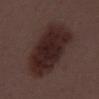Recorded during total-body skin imaging; not selected for excision or biopsy. A male subject, aged around 55. The recorded lesion diameter is about 9.5 mm. Cropped from a total-body skin-imaging series; the visible field is about 15 mm. This is a white-light tile. The lesion is on the mid back.